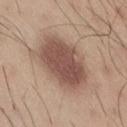follow-up: catalogued during a skin exam; not biopsied
size: ≈7 mm
patient: male, aged approximately 35
acquisition: ~15 mm tile from a whole-body skin photo
automated metrics: an average lesion color of about L≈51 a*≈19 b*≈25 (CIELAB) and a normalized lesion–skin contrast near 9.5
location: the right thigh
tile lighting: white-light illumination